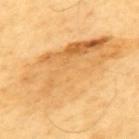Assessment:
This lesion was catalogued during total-body skin photography and was not selected for biopsy.
Background:
Measured at roughly 13 mm in maximum diameter. Located on the upper back. A male subject, aged 58 to 62. A 15 mm close-up tile from a total-body photography series done for melanoma screening. Imaged with cross-polarized lighting.